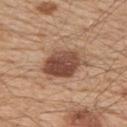Clinical impression:
Imaged during a routine full-body skin examination; the lesion was not biopsied and no histopathology is available.
Clinical summary:
This image is a 15 mm lesion crop taken from a total-body photograph. Located on the upper back. The subject is a male aged 53 to 57.